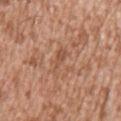Clinical impression: Recorded during total-body skin imaging; not selected for excision or biopsy. Context: A male subject, aged approximately 45. Automated image analysis of the tile measured a lesion color around L≈53 a*≈22 b*≈31 in CIELAB, roughly 7 lightness units darker than nearby skin, and a normalized border contrast of about 5. The lesion is located on the left upper arm. Longest diameter approximately 4 mm. A region of skin cropped from a whole-body photographic capture, roughly 15 mm wide.